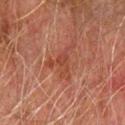The lesion was tiled from a total-body skin photograph and was not biopsied. The patient is a male in their mid-70s. The lesion is on the left forearm. The lesion-visualizer software estimated a footprint of about 6.5 mm². The analysis additionally found border irregularity of about 9.5 on a 0–10 scale, internal color variation of about 2 on a 0–10 scale, and peripheral color asymmetry of about 0.5. The software also gave a classifier nevus-likeness of about 0/100 and lesion-presence confidence of about 95/100. This image is a 15 mm lesion crop taken from a total-body photograph. This is a cross-polarized tile.